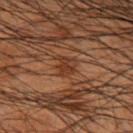Captured during whole-body skin photography for melanoma surveillance; the lesion was not biopsied.
This is a cross-polarized tile.
The lesion is located on the arm.
A roughly 15 mm field-of-view crop from a total-body skin photograph.
An algorithmic analysis of the crop reported an average lesion color of about L≈27 a*≈18 b*≈24 (CIELAB), roughly 7 lightness units darker than nearby skin, and a normalized lesion–skin contrast near 7.
About 3 mm across.
The patient is a male aged 48 to 52.